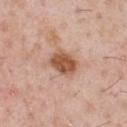Recorded during total-body skin imaging; not selected for excision or biopsy.
A region of skin cropped from a whole-body photographic capture, roughly 15 mm wide.
Longest diameter approximately 3.5 mm.
A male patient, approximately 50 years of age.
The lesion is located on the chest.
The tile uses white-light illumination.